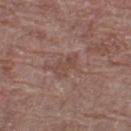{
  "biopsy_status": "not biopsied; imaged during a skin examination",
  "image": {
    "source": "total-body photography crop",
    "field_of_view_mm": 15
  },
  "site": "leg",
  "lesion_size": {
    "long_diameter_mm_approx": 3.0
  },
  "lighting": "white-light",
  "patient": {
    "sex": "female",
    "age_approx": 80
  },
  "automated_metrics": {
    "area_mm2_approx": 4.5,
    "eccentricity": 0.8,
    "shape_asymmetry": 0.35,
    "cielab_L": 46,
    "cielab_a": 19,
    "cielab_b": 24,
    "vs_skin_darker_L": 6.0,
    "border_irregularity_0_10": 4.5,
    "color_variation_0_10": 1.0
  }
}